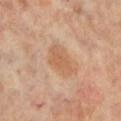Q: Is there a histopathology result?
A: imaged on a skin check; not biopsied
Q: What is the anatomic site?
A: the right leg
Q: Lesion size?
A: ~4.5 mm (longest diameter)
Q: What did automated image analysis measure?
A: about 7 CIELAB-L* units darker than the surrounding skin and a lesion-to-skin contrast of about 6 (normalized; higher = more distinct); border irregularity of about 3.5 on a 0–10 scale
Q: What lighting was used for the tile?
A: cross-polarized
Q: What kind of image is this?
A: ~15 mm crop, total-body skin-cancer survey
Q: Patient demographics?
A: female, aged around 65